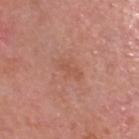Assessment: This lesion was catalogued during total-body skin photography and was not selected for biopsy. Image and clinical context: A roughly 15 mm field-of-view crop from a total-body skin photograph. A male subject aged 73 to 77. From the head or neck.